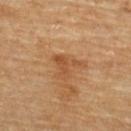Findings:
- biopsy status — imaged on a skin check; not biopsied
- acquisition — total-body-photography crop, ~15 mm field of view
- automated lesion analysis — a lesion color around L≈50 a*≈22 b*≈37 in CIELAB, a lesion–skin lightness drop of about 7, and a normalized border contrast of about 5.5; border irregularity of about 8 on a 0–10 scale, a within-lesion color-variation index near 2.5/10, and a peripheral color-asymmetry measure near 1
- subject — male, aged 83 or over
- diameter — ~3.5 mm (longest diameter)
- illumination — cross-polarized
- location — the upper back